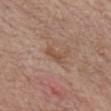biopsy status: imaged on a skin check; not biopsied | anatomic site: the chest | lighting: white-light illumination | lesion diameter: ~2.5 mm (longest diameter) | image: 15 mm crop, total-body photography | subject: male, in their 70s.